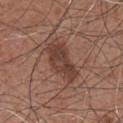This lesion was catalogued during total-body skin photography and was not selected for biopsy.
Approximately 5.5 mm at its widest.
Automated image analysis of the tile measured an area of roughly 11 mm² and an eccentricity of roughly 0.9. The analysis additionally found a lesion color around L≈40 a*≈20 b*≈25 in CIELAB, about 10 CIELAB-L* units darker than the surrounding skin, and a normalized border contrast of about 8. The software also gave a color-variation rating of about 3.5/10 and radial color variation of about 1.
A male subject aged 73 to 77.
A 15 mm crop from a total-body photograph taken for skin-cancer surveillance.
The tile uses white-light illumination.
The lesion is located on the chest.